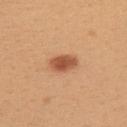Q: Was this lesion biopsied?
A: total-body-photography surveillance lesion; no biopsy
Q: Where on the body is the lesion?
A: the upper back
Q: What kind of image is this?
A: total-body-photography crop, ~15 mm field of view
Q: Patient demographics?
A: female, in their mid-20s